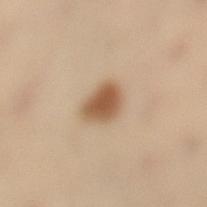biopsy status: total-body-photography surveillance lesion; no biopsy
automated lesion analysis: an average lesion color of about L≈46 a*≈16 b*≈29 (CIELAB), a lesion–skin lightness drop of about 12, and a normalized lesion–skin contrast near 9.5
location: the left lower leg
imaging modality: ~15 mm crop, total-body skin-cancer survey
lesion size: ~3.5 mm (longest diameter)
patient: female, aged approximately 50